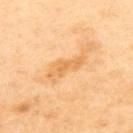Captured during whole-body skin photography for melanoma surveillance; the lesion was not biopsied.
From the upper back.
Cropped from a whole-body photographic skin survey; the tile spans about 15 mm.
The subject is a female aged 38–42.